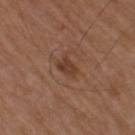Imaged during a routine full-body skin examination; the lesion was not biopsied and no histopathology is available. Imaged with white-light lighting. A male subject aged approximately 75. An algorithmic analysis of the crop reported a mean CIELAB color near L≈38 a*≈21 b*≈28, a lesion–skin lightness drop of about 8, and a normalized border contrast of about 7. The analysis additionally found a border-irregularity rating of about 2.5/10, a within-lesion color-variation index near 2/10, and a peripheral color-asymmetry measure near 0.5. From the arm. This image is a 15 mm lesion crop taken from a total-body photograph. Longest diameter approximately 2.5 mm.